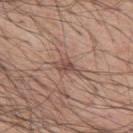notes: total-body-photography surveillance lesion; no biopsy
body site: the mid back
tile lighting: white-light
lesion diameter: ~3 mm (longest diameter)
subject: male, about 55 years old
image source: ~15 mm tile from a whole-body skin photo